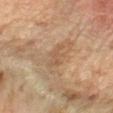Impression: This lesion was catalogued during total-body skin photography and was not selected for biopsy. Context: A female subject about 60 years old. A region of skin cropped from a whole-body photographic capture, roughly 15 mm wide. From the left forearm.